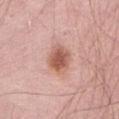notes=no biopsy performed (imaged during a skin exam); subject=female, roughly 65 years of age; site=the abdomen; image source=~15 mm crop, total-body skin-cancer survey.